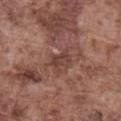workup: total-body-photography surveillance lesion; no biopsy | subject: male, in their mid-70s | image source: ~15 mm crop, total-body skin-cancer survey | body site: the abdomen | image-analysis metrics: an area of roughly 5 mm², an outline eccentricity of about 0.7 (0 = round, 1 = elongated), and two-axis asymmetry of about 0.25; a mean CIELAB color near L≈41 a*≈21 b*≈23, about 7 CIELAB-L* units darker than the surrounding skin, and a normalized lesion–skin contrast near 6 | lesion size: ~3 mm (longest diameter) | illumination: white-light illumination.